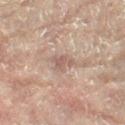Part of a total-body skin-imaging series; this lesion was reviewed on a skin check and was not flagged for biopsy.